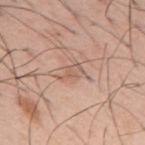<case>
<biopsy_status>not biopsied; imaged during a skin examination</biopsy_status>
<image>
  <source>total-body photography crop</source>
  <field_of_view_mm>15</field_of_view_mm>
</image>
<automated_metrics>
  <area_mm2_approx>3.5</area_mm2_approx>
  <eccentricity>0.8</eccentricity>
  <shape_asymmetry>0.4</shape_asymmetry>
  <cielab_L>59</cielab_L>
  <cielab_a>19</cielab_a>
  <cielab_b>28</cielab_b>
  <vs_skin_darker_L>8.0</vs_skin_darker_L>
  <vs_skin_contrast_norm>5.0</vs_skin_contrast_norm>
</automated_metrics>
<lighting>white-light</lighting>
<patient>
  <sex>male</sex>
  <age_approx>55</age_approx>
</patient>
<site>mid back</site>
</case>